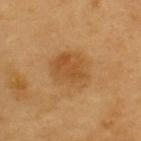Impression:
The lesion was photographed on a routine skin check and not biopsied; there is no pathology result.
Image and clinical context:
A close-up tile cropped from a whole-body skin photograph, about 15 mm across. Automated tile analysis of the lesion measured a mean CIELAB color near L≈51 a*≈20 b*≈41 and roughly 8 lightness units darker than nearby skin. And it measured a border-irregularity index near 2.5/10, a within-lesion color-variation index near 3.5/10, and peripheral color asymmetry of about 1. It also reported an automated nevus-likeness rating near 90 out of 100. A male subject, aged 58 to 62. This is a cross-polarized tile. On the back. About 5 mm across.